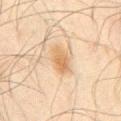| field | value |
|---|---|
| biopsy status | total-body-photography surveillance lesion; no biopsy |
| illumination | cross-polarized illumination |
| body site | the abdomen |
| subject | male, about 45 years old |
| diameter | about 3.5 mm |
| image source | ~15 mm crop, total-body skin-cancer survey |